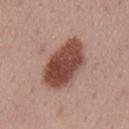Q: Was a biopsy performed?
A: total-body-photography surveillance lesion; no biopsy
Q: What kind of image is this?
A: ~15 mm tile from a whole-body skin photo
Q: What lighting was used for the tile?
A: white-light
Q: Where on the body is the lesion?
A: the mid back
Q: What are the patient's age and sex?
A: male, aged around 55